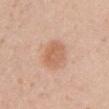| field | value |
|---|---|
| location | the right upper arm |
| imaging modality | ~15 mm tile from a whole-body skin photo |
| lesion diameter | about 3.5 mm |
| tile lighting | white-light |
| patient | female, aged around 20 |